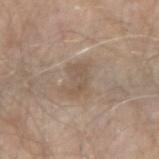notes — imaged on a skin check; not biopsied | subject — male, aged around 65 | location — the right forearm | image — ~15 mm tile from a whole-body skin photo.